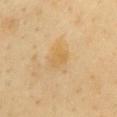The lesion was photographed on a routine skin check and not biopsied; there is no pathology result. Located on the front of the torso. Automated image analysis of the tile measured an area of roughly 5.5 mm², an outline eccentricity of about 0.6 (0 = round, 1 = elongated), and two-axis asymmetry of about 0.25. The analysis additionally found a classifier nevus-likeness of about 0/100. A female patient, approximately 50 years of age. Approximately 3 mm at its widest. This image is a 15 mm lesion crop taken from a total-body photograph. Imaged with cross-polarized lighting.